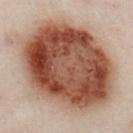| field | value |
|---|---|
| automated lesion analysis | a lesion area of about 95 mm², an eccentricity of roughly 0.65, and a symmetry-axis asymmetry near 0.15; about 16 CIELAB-L* units darker than the surrounding skin and a normalized border contrast of about 13; a within-lesion color-variation index near 8.5/10 |
| location | the left leg |
| patient | male, aged 48–52 |
| imaging modality | total-body-photography crop, ~15 mm field of view |
| tile lighting | cross-polarized illumination |